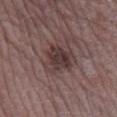An algorithmic analysis of the crop reported an area of roughly 11 mm², an eccentricity of roughly 0.5, and two-axis asymmetry of about 0.3. The analysis additionally found roughly 9 lightness units darker than nearby skin and a normalized border contrast of about 8. The lesion is located on the right lower leg. A roughly 15 mm field-of-view crop from a total-body skin photograph. The subject is a male aged around 75. This is a white-light tile. Measured at roughly 4 mm in maximum diameter.